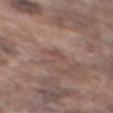Recorded during total-body skin imaging; not selected for excision or biopsy. Located on the back. The subject is a male aged 73–77. The tile uses white-light illumination. Longest diameter approximately 2.5 mm. A lesion tile, about 15 mm wide, cut from a 3D total-body photograph. The total-body-photography lesion software estimated a lesion area of about 3 mm², an eccentricity of roughly 0.85, and a symmetry-axis asymmetry near 0.3. The analysis additionally found a mean CIELAB color near L≈49 a*≈18 b*≈23, roughly 6 lightness units darker than nearby skin, and a normalized border contrast of about 5.